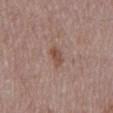This lesion was catalogued during total-body skin photography and was not selected for biopsy.
Approximately 3 mm at its widest.
Captured under white-light illumination.
A 15 mm crop from a total-body photograph taken for skin-cancer surveillance.
A male subject, approximately 75 years of age.
From the mid back.